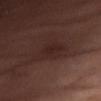The lesion was tiled from a total-body skin photograph and was not biopsied. About 4.5 mm across. A region of skin cropped from a whole-body photographic capture, roughly 15 mm wide. This is a cross-polarized tile. On the abdomen. A female patient aged 53–57.